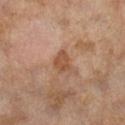Part of a total-body skin-imaging series; this lesion was reviewed on a skin check and was not flagged for biopsy. Captured under cross-polarized illumination. From the right lower leg. Cropped from a whole-body photographic skin survey; the tile spans about 15 mm. Longest diameter approximately 2.5 mm. A female subject, aged around 60.